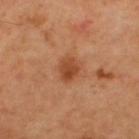Findings:
* biopsy status: total-body-photography surveillance lesion; no biopsy
* image source: 15 mm crop, total-body photography
* size: about 3 mm
* tile lighting: cross-polarized
* subject: male, about 60 years old
* site: the upper back